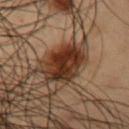Impression: The lesion was photographed on a routine skin check and not biopsied; there is no pathology result. Image and clinical context: Cropped from a total-body skin-imaging series; the visible field is about 15 mm. From the chest. A male subject in their mid-50s.